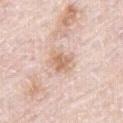Imaged during a routine full-body skin examination; the lesion was not biopsied and no histopathology is available.